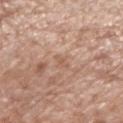notes: no biopsy performed (imaged during a skin exam) | subject: male, approximately 75 years of age | image source: 15 mm crop, total-body photography | automated lesion analysis: an average lesion color of about L≈57 a*≈19 b*≈30 (CIELAB); a within-lesion color-variation index near 0/10 and peripheral color asymmetry of about 0; a classifier nevus-likeness of about 0/100 and lesion-presence confidence of about 75/100 | diameter: ~2.5 mm (longest diameter) | illumination: white-light illumination | site: the left forearm.